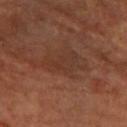Measured at roughly 5 mm in maximum diameter.
A female subject in their mid- to late 60s.
This image is a 15 mm lesion crop taken from a total-body photograph.
From the left forearm.
The lesion-visualizer software estimated a footprint of about 9.5 mm² and a shape-asymmetry score of about 0.5 (0 = symmetric). The software also gave a lesion-detection confidence of about 95/100.